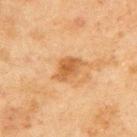Recorded during total-body skin imaging; not selected for excision or biopsy. Located on the back. Measured at roughly 3.5 mm in maximum diameter. The tile uses cross-polarized illumination. A male patient about 45 years old. A close-up tile cropped from a whole-body skin photograph, about 15 mm across. The lesion-visualizer software estimated an area of roughly 6.5 mm². The analysis additionally found a border-irregularity index near 2.5/10 and a within-lesion color-variation index near 3/10. It also reported a nevus-likeness score of about 45/100 and a lesion-detection confidence of about 100/100.